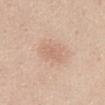Q: Was a biopsy performed?
A: no biopsy performed (imaged during a skin exam)
Q: How was the tile lit?
A: white-light
Q: Where on the body is the lesion?
A: the abdomen
Q: How was this image acquired?
A: 15 mm crop, total-body photography
Q: Patient demographics?
A: female, aged approximately 45